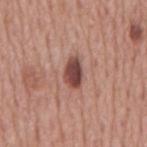biopsy status: imaged on a skin check; not biopsied.